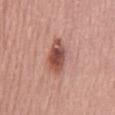Impression:
The lesion was tiled from a total-body skin photograph and was not biopsied.
Acquisition and patient details:
Automated tile analysis of the lesion measured an average lesion color of about L≈51 a*≈26 b*≈26 (CIELAB), about 14 CIELAB-L* units darker than the surrounding skin, and a lesion-to-skin contrast of about 9.5 (normalized; higher = more distinct). The analysis additionally found a within-lesion color-variation index near 7/10 and a peripheral color-asymmetry measure near 2. The lesion is located on the abdomen. Longest diameter approximately 5 mm. The subject is a male aged around 75. A 15 mm close-up tile from a total-body photography series done for melanoma screening. The tile uses white-light illumination.